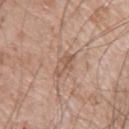Notes:
– follow-up · no biopsy performed (imaged during a skin exam)
– image · ~15 mm crop, total-body skin-cancer survey
– site · the arm
– lesion diameter · about 3 mm
– lighting · white-light illumination
– TBP lesion metrics · a lesion color around L≈56 a*≈18 b*≈29 in CIELAB and a normalized border contrast of about 5.5
– patient · male, approximately 75 years of age